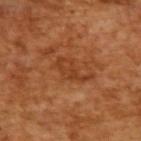The total-body-photography lesion software estimated border irregularity of about 6 on a 0–10 scale, a color-variation rating of about 2/10, and radial color variation of about 0.5.
The patient is a male aged approximately 65.
Cropped from a whole-body photographic skin survey; the tile spans about 15 mm.
Imaged with cross-polarized lighting.
Longest diameter approximately 4 mm.